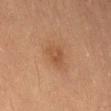Case summary:
– subject — male, roughly 55 years of age
– acquisition — 15 mm crop, total-body photography
– body site — the lower back